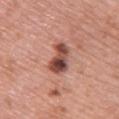* workup · catalogued during a skin exam; not biopsied
* anatomic site · the upper back
* size · ≈4 mm
* acquisition · ~15 mm crop, total-body skin-cancer survey
* image-analysis metrics · an outline eccentricity of about 0.8 (0 = round, 1 = elongated) and a symmetry-axis asymmetry near 0.3; a classifier nevus-likeness of about 30/100
* lighting · white-light illumination
* subject · female, roughly 60 years of age